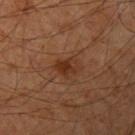notes — no biopsy performed (imaged during a skin exam)
image-analysis metrics — an area of roughly 5 mm², an outline eccentricity of about 0.7 (0 = round, 1 = elongated), and two-axis asymmetry of about 0.25; a lesion color around L≈29 a*≈20 b*≈28 in CIELAB, roughly 7 lightness units darker than nearby skin, and a normalized lesion–skin contrast near 7.5; border irregularity of about 2.5 on a 0–10 scale, a within-lesion color-variation index near 3/10, and peripheral color asymmetry of about 1
lighting — cross-polarized
image source — 15 mm crop, total-body photography
diameter — ~3 mm (longest diameter)
location — the right upper arm
patient — male, roughly 60 years of age